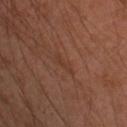location — the arm
size — about 3 mm
automated metrics — a color-variation rating of about 0/10 and a peripheral color-asymmetry measure near 0; a classifier nevus-likeness of about 0/100 and a detector confidence of about 80 out of 100 that the crop contains a lesion
patient — male, aged 43 to 47
lighting — cross-polarized illumination
acquisition — ~15 mm crop, total-body skin-cancer survey